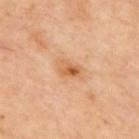Recorded during total-body skin imaging; not selected for excision or biopsy.
This is a cross-polarized tile.
Longest diameter approximately 3 mm.
Cropped from a total-body skin-imaging series; the visible field is about 15 mm.
From the back.